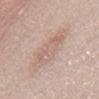{"automated_metrics": {"area_mm2_approx": 15.0, "eccentricity": 0.85, "shape_asymmetry": 0.2, "cielab_L": 63, "cielab_a": 17, "cielab_b": 25, "vs_skin_darker_L": 7.0, "vs_skin_contrast_norm": 4.5, "nevus_likeness_0_100": 0}, "patient": {"sex": "female", "age_approx": 50}, "lesion_size": {"long_diameter_mm_approx": 6.0}, "site": "chest", "image": {"source": "total-body photography crop", "field_of_view_mm": 15}, "lighting": "white-light"}